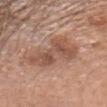Imaged during a routine full-body skin examination; the lesion was not biopsied and no histopathology is available. Imaged with white-light lighting. About 7 mm across. A 15 mm crop from a total-body photograph taken for skin-cancer surveillance. A female subject, in their mid-60s. The lesion is on the head or neck.